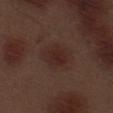Captured during whole-body skin photography for melanoma surveillance; the lesion was not biopsied. A male patient, aged around 70. Measured at roughly 4 mm in maximum diameter. The lesion is on the left thigh. Cropped from a whole-body photographic skin survey; the tile spans about 15 mm.